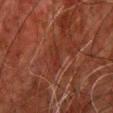  biopsy_status: not biopsied; imaged during a skin examination
  image:
    source: total-body photography crop
    field_of_view_mm: 15
  lesion_size:
    long_diameter_mm_approx: 3.5
  patient:
    sex: male
    age_approx: 60
  site: chest
  lighting: cross-polarized